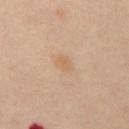Q: Was this lesion biopsied?
A: catalogued during a skin exam; not biopsied
Q: Patient demographics?
A: male, aged around 50
Q: What kind of image is this?
A: total-body-photography crop, ~15 mm field of view
Q: Where on the body is the lesion?
A: the abdomen